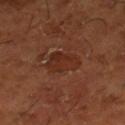The lesion was tiled from a total-body skin photograph and was not biopsied. Located on the right lower leg. A male subject aged approximately 65. This image is a 15 mm lesion crop taken from a total-body photograph.